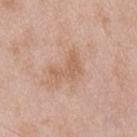{"biopsy_status": "not biopsied; imaged during a skin examination", "image": {"source": "total-body photography crop", "field_of_view_mm": 15}, "automated_metrics": {"eccentricity": 0.8, "shape_asymmetry": 0.6, "color_variation_0_10": 1.5, "lesion_detection_confidence_0_100": 100}, "lighting": "white-light", "site": "chest", "patient": {"sex": "male", "age_approx": 50}}